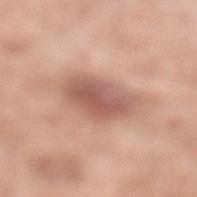Recorded during total-body skin imaging; not selected for excision or biopsy. An algorithmic analysis of the crop reported a lesion area of about 15 mm² and an outline eccentricity of about 0.8 (0 = round, 1 = elongated). The software also gave roughly 12 lightness units darker than nearby skin. The software also gave a border-irregularity rating of about 2.5/10 and a color-variation rating of about 4.5/10. It also reported a detector confidence of about 100 out of 100 that the crop contains a lesion. The lesion is located on the left lower leg. A 15 mm crop from a total-body photograph taken for skin-cancer surveillance. The patient is a male about 80 years old. About 5.5 mm across. This is a white-light tile.